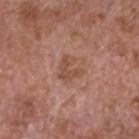<tbp_lesion>
<biopsy_status>not biopsied; imaged during a skin examination</biopsy_status>
<image>
  <source>total-body photography crop</source>
  <field_of_view_mm>15</field_of_view_mm>
</image>
<patient>
  <sex>male</sex>
  <age_approx>75</age_approx>
</patient>
<lesion_size>
  <long_diameter_mm_approx>3.0</long_diameter_mm_approx>
</lesion_size>
<site>head or neck</site>
</tbp_lesion>